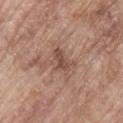The lesion was photographed on a routine skin check and not biopsied; there is no pathology result. From the upper back. A male subject, roughly 65 years of age. This is a white-light tile. Measured at roughly 3.5 mm in maximum diameter. An algorithmic analysis of the crop reported a footprint of about 5.5 mm², a shape eccentricity near 0.8, and a shape-asymmetry score of about 0.4 (0 = symmetric). And it measured an average lesion color of about L≈49 a*≈19 b*≈25 (CIELAB), roughly 9 lightness units darker than nearby skin, and a normalized lesion–skin contrast near 6.5. And it measured a border-irregularity rating of about 5.5/10, a within-lesion color-variation index near 2/10, and peripheral color asymmetry of about 0.5. The analysis additionally found an automated nevus-likeness rating near 0 out of 100 and a lesion-detection confidence of about 100/100. A lesion tile, about 15 mm wide, cut from a 3D total-body photograph.